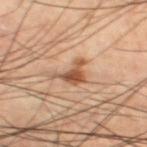The lesion was tiled from a total-body skin photograph and was not biopsied. An algorithmic analysis of the crop reported an automated nevus-likeness rating near 90 out of 100. Imaged with cross-polarized lighting. A male patient aged 58–62. The lesion is located on the left thigh. A 15 mm close-up tile from a total-body photography series done for melanoma screening.